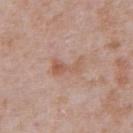No biopsy was performed on this lesion — it was imaged during a full skin examination and was not determined to be concerning. A male subject, aged 63–67. A 15 mm crop from a total-body photograph taken for skin-cancer surveillance. The lesion is on the abdomen.